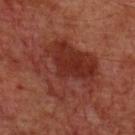Q: Was a biopsy performed?
A: no biopsy performed (imaged during a skin exam)
Q: What is the lesion's diameter?
A: about 8.5 mm
Q: What is the anatomic site?
A: the chest
Q: Who is the patient?
A: male, aged approximately 60
Q: What lighting was used for the tile?
A: cross-polarized
Q: What is the imaging modality?
A: ~15 mm tile from a whole-body skin photo